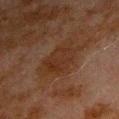| key | value |
|---|---|
| follow-up | no biopsy performed (imaged during a skin exam) |
| body site | the left upper arm |
| subject | male, about 80 years old |
| tile lighting | cross-polarized illumination |
| imaging modality | 15 mm crop, total-body photography |
| image-analysis metrics | an area of roughly 14 mm², a shape eccentricity near 0.75, and a symmetry-axis asymmetry near 0.25; a border-irregularity index near 3.5/10, a within-lesion color-variation index near 2.5/10, and peripheral color asymmetry of about 1 |